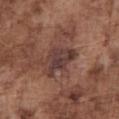<tbp_lesion>
<biopsy_status>not biopsied; imaged during a skin examination</biopsy_status>
<patient>
  <sex>male</sex>
  <age_approx>75</age_approx>
</patient>
<image>
  <source>total-body photography crop</source>
  <field_of_view_mm>15</field_of_view_mm>
</image>
<lighting>white-light</lighting>
<automated_metrics>
  <area_mm2_approx>9.5</area_mm2_approx>
  <eccentricity>0.75</eccentricity>
  <shape_asymmetry>0.45</shape_asymmetry>
  <border_irregularity_0_10>4.5</border_irregularity_0_10>
  <color_variation_0_10>3.5</color_variation_0_10>
  <peripheral_color_asymmetry>1.0</peripheral_color_asymmetry>
</automated_metrics>
<site>chest</site>
<lesion_size>
  <long_diameter_mm_approx>4.5</long_diameter_mm_approx>
</lesion_size>
</tbp_lesion>